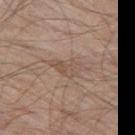Findings:
- notes: total-body-photography surveillance lesion; no biopsy
- image: 15 mm crop, total-body photography
- lighting: white-light illumination
- TBP lesion metrics: roughly 7 lightness units darker than nearby skin; a within-lesion color-variation index near 2.5/10; a classifier nevus-likeness of about 0/100 and a lesion-detection confidence of about 90/100
- size: about 4.5 mm
- patient: male, aged approximately 60
- location: the left thigh